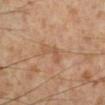Impression:
This lesion was catalogued during total-body skin photography and was not selected for biopsy.
Background:
Approximately 3.5 mm at its widest. Captured under cross-polarized illumination. A 15 mm crop from a total-body photograph taken for skin-cancer surveillance. The total-body-photography lesion software estimated a shape eccentricity near 0.85 and a shape-asymmetry score of about 0.4 (0 = symmetric). The software also gave a lesion color around L≈55 a*≈20 b*≈34 in CIELAB, about 6 CIELAB-L* units darker than the surrounding skin, and a normalized lesion–skin contrast near 5. A male patient aged 53–57. On the left lower leg.